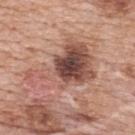Part of a total-body skin-imaging series; this lesion was reviewed on a skin check and was not flagged for biopsy. The total-body-photography lesion software estimated a lesion area of about 25 mm², an outline eccentricity of about 0.8 (0 = round, 1 = elongated), and a symmetry-axis asymmetry near 0.55. And it measured a normalized border contrast of about 10. And it measured a border-irregularity index near 7/10, internal color variation of about 9.5 on a 0–10 scale, and a peripheral color-asymmetry measure near 3. And it measured a nevus-likeness score of about 45/100. A female patient, aged 58 to 62. Cropped from a total-body skin-imaging series; the visible field is about 15 mm. On the upper back. Approximately 8 mm at its widest.